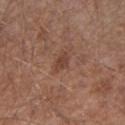Q: Was a biopsy performed?
A: imaged on a skin check; not biopsied
Q: Illumination type?
A: white-light illumination
Q: What kind of image is this?
A: 15 mm crop, total-body photography
Q: What is the anatomic site?
A: the right upper arm
Q: Automated lesion metrics?
A: an average lesion color of about L≈42 a*≈21 b*≈26 (CIELAB), roughly 8 lightness units darker than nearby skin, and a normalized border contrast of about 6.5; border irregularity of about 3 on a 0–10 scale and peripheral color asymmetry of about 0.5; an automated nevus-likeness rating near 40 out of 100 and lesion-presence confidence of about 100/100
Q: What are the patient's age and sex?
A: male, roughly 55 years of age